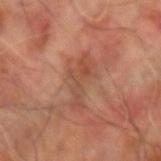<record>
<lesion_size>
  <long_diameter_mm_approx>5.5</long_diameter_mm_approx>
</lesion_size>
<image>
  <source>total-body photography crop</source>
  <field_of_view_mm>15</field_of_view_mm>
</image>
<lighting>cross-polarized</lighting>
<patient>
  <sex>male</sex>
  <age_approx>60</age_approx>
</patient>
<automated_metrics>
  <border_irregularity_0_10>7.5</border_irregularity_0_10>
  <peripheral_color_asymmetry>1.0</peripheral_color_asymmetry>
  <lesion_detection_confidence_0_100>75</lesion_detection_confidence_0_100>
</automated_metrics>
<site>right arm</site>
</record>